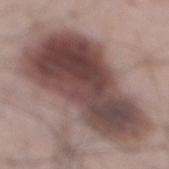This lesion was catalogued during total-body skin photography and was not selected for biopsy. The recorded lesion diameter is about 13.5 mm. The patient is a male aged 63 to 67. Imaged with white-light lighting. On the back. A roughly 15 mm field-of-view crop from a total-body skin photograph.